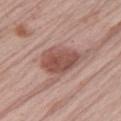Part of a total-body skin-imaging series; this lesion was reviewed on a skin check and was not flagged for biopsy. A male subject approximately 65 years of age. A 15 mm close-up tile from a total-body photography series done for melanoma screening. The tile uses white-light illumination. Automated tile analysis of the lesion measured an area of roughly 15 mm², a shape eccentricity near 0.6, and a symmetry-axis asymmetry near 0.2. And it measured a mean CIELAB color near L≈51 a*≈22 b*≈24 and a lesion–skin lightness drop of about 12. The analysis additionally found a border-irregularity rating of about 2.5/10, a color-variation rating of about 4/10, and peripheral color asymmetry of about 1.5. It also reported a nevus-likeness score of about 45/100 and lesion-presence confidence of about 100/100. Measured at roughly 5 mm in maximum diameter. From the left upper arm.